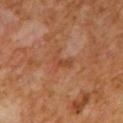<lesion>
  <biopsy_status>not biopsied; imaged during a skin examination</biopsy_status>
  <lighting>cross-polarized</lighting>
  <automated_metrics>
    <cielab_L>43</cielab_L>
    <cielab_a>24</cielab_a>
    <cielab_b>34</cielab_b>
    <vs_skin_darker_L>6.0</vs_skin_darker_L>
    <vs_skin_contrast_norm>5.5</vs_skin_contrast_norm>
  </automated_metrics>
  <patient>
    <sex>male</sex>
    <age_approx>65</age_approx>
  </patient>
  <site>back</site>
  <image>
    <source>total-body photography crop</source>
    <field_of_view_mm>15</field_of_view_mm>
  </image>
</lesion>